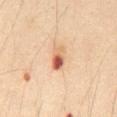Recorded during total-body skin imaging; not selected for excision or biopsy. This is a cross-polarized tile. A 15 mm close-up tile from a total-body photography series done for melanoma screening. Automated tile analysis of the lesion measured an automated nevus-likeness rating near 0 out of 100 and lesion-presence confidence of about 100/100. Approximately 3 mm at its widest. A male subject aged approximately 65. From the abdomen.